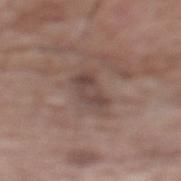workup = no biopsy performed (imaged during a skin exam)
site = the back
lesion size = ≈4 mm
subject = male, aged 58–62
lighting = white-light illumination
acquisition = 15 mm crop, total-body photography